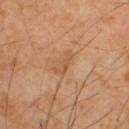<lesion>
  <biopsy_status>not biopsied; imaged during a skin examination</biopsy_status>
  <image>
    <source>total-body photography crop</source>
    <field_of_view_mm>15</field_of_view_mm>
  </image>
  <lesion_size>
    <long_diameter_mm_approx>3.0</long_diameter_mm_approx>
  </lesion_size>
  <site>upper back</site>
  <lighting>cross-polarized</lighting>
  <patient>
    <sex>male</sex>
    <age_approx>50</age_approx>
  </patient>
  <automated_metrics>
    <area_mm2_approx>3.5</area_mm2_approx>
    <shape_asymmetry>0.5</shape_asymmetry>
  </automated_metrics>
</lesion>